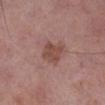The lesion was tiled from a total-body skin photograph and was not biopsied.
A male patient aged approximately 70.
A 15 mm close-up extracted from a 3D total-body photography capture.
The lesion is on the right lower leg.
Longest diameter approximately 3.5 mm.